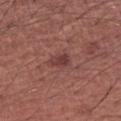biopsy_status: not biopsied; imaged during a skin examination
site: right forearm
automated_metrics:
  area_mm2_approx: 4.0
  eccentricity: 0.8
  shape_asymmetry: 0.2
  border_irregularity_0_10: 2.0
  peripheral_color_asymmetry: 1.0
image:
  source: total-body photography crop
  field_of_view_mm: 15
patient:
  sex: male
  age_approx: 45
lighting: white-light
lesion_size:
  long_diameter_mm_approx: 2.5